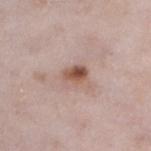Q: Is there a histopathology result?
A: no biopsy performed (imaged during a skin exam)
Q: Where on the body is the lesion?
A: the leg
Q: What is the imaging modality?
A: ~15 mm tile from a whole-body skin photo
Q: Lesion size?
A: ≈3 mm
Q: How was the tile lit?
A: white-light
Q: Who is the patient?
A: female, aged 28–32